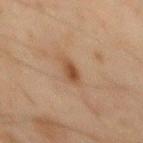workup = total-body-photography surveillance lesion; no biopsy | anatomic site = the mid back | illumination = cross-polarized illumination | acquisition = ~15 mm tile from a whole-body skin photo | patient = male, aged 43–47.